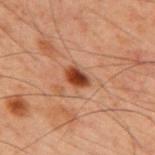  biopsy_status: not biopsied; imaged during a skin examination
  patient:
    sex: male
    age_approx: 60
  automated_metrics:
    eccentricity: 0.75
    shape_asymmetry: 0.25
    cielab_L: 32
    cielab_a: 23
    cielab_b: 29
    vs_skin_darker_L: 14.0
    vs_skin_contrast_norm: 12.5
    nevus_likeness_0_100: 100
  lighting: cross-polarized
  image:
    source: total-body photography crop
    field_of_view_mm: 15
  lesion_size:
    long_diameter_mm_approx: 2.5
  site: back